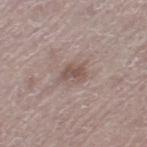Notes:
• biopsy status: imaged on a skin check; not biopsied
• site: the left thigh
• image: ~15 mm crop, total-body skin-cancer survey
• lesion diameter: ~3 mm (longest diameter)
• patient: female, aged 63 to 67